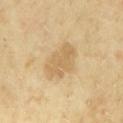The lesion is located on the chest. This image is a 15 mm lesion crop taken from a total-body photograph. A male subject, aged around 65.